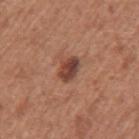notes: imaged on a skin check; not biopsied | anatomic site: the left upper arm | image-analysis metrics: an area of roughly 5 mm² and a symmetry-axis asymmetry near 0.25; a border-irregularity rating of about 2/10, a color-variation rating of about 4.5/10, and radial color variation of about 1.5 | patient: female, in their 50s | image: total-body-photography crop, ~15 mm field of view.